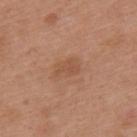anatomic site: the upper back
subject: female, about 40 years old
image source: total-body-photography crop, ~15 mm field of view